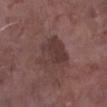Acquisition and patient details: Captured under white-light illumination. A lesion tile, about 15 mm wide, cut from a 3D total-body photograph. The recorded lesion diameter is about 4.5 mm. The lesion is on the right lower leg. A male patient, roughly 75 years of age.